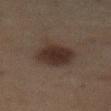Case summary:
– acquisition — total-body-photography crop, ~15 mm field of view
– patient — male, about 75 years old
– tile lighting — cross-polarized
– lesion diameter — ~4.5 mm (longest diameter)
– automated metrics — a normalized lesion–skin contrast near 9.5; an automated nevus-likeness rating near 85 out of 100 and a detector confidence of about 100 out of 100 that the crop contains a lesion
– anatomic site — the abdomen